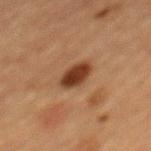{
  "biopsy_status": "not biopsied; imaged during a skin examination",
  "automated_metrics": {
    "area_mm2_approx": 6.5,
    "eccentricity": 0.6,
    "shape_asymmetry": 0.15,
    "nevus_likeness_0_100": 100,
    "lesion_detection_confidence_0_100": 100
  },
  "lesion_size": {
    "long_diameter_mm_approx": 3.0
  },
  "image": {
    "source": "total-body photography crop",
    "field_of_view_mm": 15
  },
  "patient": {
    "sex": "female",
    "age_approx": 70
  },
  "lighting": "cross-polarized",
  "site": "mid back"
}